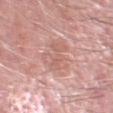biopsy status: no biopsy performed (imaged during a skin exam) | site: the head or neck | automated metrics: an outline eccentricity of about 0.75 (0 = round, 1 = elongated); internal color variation of about 3.5 on a 0–10 scale and a peripheral color-asymmetry measure near 1 | lesion diameter: ≈5 mm | subject: male, aged around 70 | image: 15 mm crop, total-body photography.